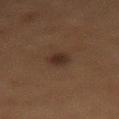The lesion was photographed on a routine skin check and not biopsied; there is no pathology result. On the back. A female patient, aged approximately 50. A region of skin cropped from a whole-body photographic capture, roughly 15 mm wide. Measured at roughly 3 mm in maximum diameter.